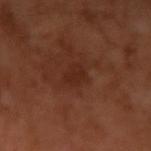Image and clinical context: A male subject approximately 55 years of age. On the arm. A roughly 15 mm field-of-view crop from a total-body skin photograph. This is a cross-polarized tile. Approximately 2.5 mm at its widest.